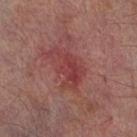Captured during whole-body skin photography for melanoma surveillance; the lesion was not biopsied.
The lesion is on the right lower leg.
A lesion tile, about 15 mm wide, cut from a 3D total-body photograph.
A male subject, in their mid- to late 60s.